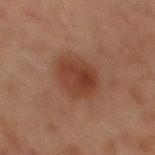This lesion was catalogued during total-body skin photography and was not selected for biopsy. Captured under cross-polarized illumination. A male patient, in their mid-60s. Automated image analysis of the tile measured a lesion area of about 14 mm², an outline eccentricity of about 0.7 (0 = round, 1 = elongated), and a symmetry-axis asymmetry near 0.15. It also reported a lesion color around L≈31 a*≈19 b*≈24 in CIELAB and a normalized border contrast of about 7.5. The analysis additionally found a classifier nevus-likeness of about 80/100 and a detector confidence of about 100 out of 100 that the crop contains a lesion. Cropped from a whole-body photographic skin survey; the tile spans about 15 mm. The lesion is located on the front of the torso.